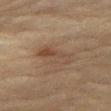notes: catalogued during a skin exam; not biopsied | anatomic site: the right thigh | lesion diameter: ≈5 mm | patient: female, approximately 80 years of age | acquisition: 15 mm crop, total-body photography | image-analysis metrics: an area of roughly 7.5 mm², an outline eccentricity of about 0.95 (0 = round, 1 = elongated), and a symmetry-axis asymmetry near 0.2; a border-irregularity rating of about 3/10, a within-lesion color-variation index near 7.5/10, and peripheral color asymmetry of about 2.5.